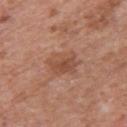Notes:
- notes — no biopsy performed (imaged during a skin exam)
- body site — the left upper arm
- tile lighting — white-light illumination
- automated lesion analysis — a lesion area of about 8 mm² and an outline eccentricity of about 0.55 (0 = round, 1 = elongated); a mean CIELAB color near L≈49 a*≈23 b*≈30, roughly 8 lightness units darker than nearby skin, and a lesion-to-skin contrast of about 6 (normalized; higher = more distinct); a border-irregularity rating of about 2.5/10, a within-lesion color-variation index near 3.5/10, and peripheral color asymmetry of about 1; an automated nevus-likeness rating near 10 out of 100
- diameter — about 3.5 mm
- patient — male, in their mid- to late 60s
- imaging modality — 15 mm crop, total-body photography